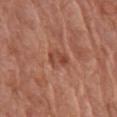Part of a total-body skin-imaging series; this lesion was reviewed on a skin check and was not flagged for biopsy.
The lesion is on the right upper arm.
The lesion's longest dimension is about 3 mm.
The tile uses white-light illumination.
This image is a 15 mm lesion crop taken from a total-body photograph.
A female patient roughly 80 years of age.